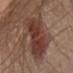Impression: The lesion was photographed on a routine skin check and not biopsied; there is no pathology result. Acquisition and patient details: A close-up tile cropped from a whole-body skin photograph, about 15 mm across. Captured under white-light illumination. A male subject, aged 63–67. The lesion is located on the chest. Measured at roughly 8.5 mm in maximum diameter.